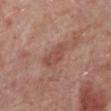No biopsy was performed on this lesion — it was imaged during a full skin examination and was not determined to be concerning. Measured at roughly 4 mm in maximum diameter. Cropped from a total-body skin-imaging series; the visible field is about 15 mm. The lesion is located on the right lower leg. A male patient in their 70s.